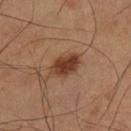No biopsy was performed on this lesion — it was imaged during a full skin examination and was not determined to be concerning. From the leg. This image is a 15 mm lesion crop taken from a total-body photograph. A male subject, aged 58 to 62.